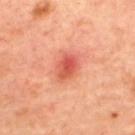follow-up — total-body-photography surveillance lesion; no biopsy
image-analysis metrics — internal color variation of about 5.5 on a 0–10 scale; a nevus-likeness score of about 10/100 and lesion-presence confidence of about 100/100
anatomic site — the back
subject — female, in their mid-40s
lighting — cross-polarized
acquisition — ~15 mm tile from a whole-body skin photo